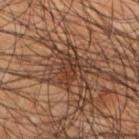The lesion was photographed on a routine skin check and not biopsied; there is no pathology result. The tile uses cross-polarized illumination. A lesion tile, about 15 mm wide, cut from a 3D total-body photograph. The recorded lesion diameter is about 3.5 mm. The lesion is on the left lower leg. A male patient aged around 45.